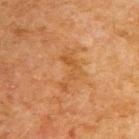Assessment:
Imaged during a routine full-body skin examination; the lesion was not biopsied and no histopathology is available.
Context:
A male subject, approximately 50 years of age. Cropped from a whole-body photographic skin survey; the tile spans about 15 mm. This is a cross-polarized tile. From the upper back. An algorithmic analysis of the crop reported an area of roughly 7.5 mm², an outline eccentricity of about 0.9 (0 = round, 1 = elongated), and two-axis asymmetry of about 0.65. It also reported a mean CIELAB color near L≈44 a*≈21 b*≈36 and about 5 CIELAB-L* units darker than the surrounding skin. It also reported an automated nevus-likeness rating near 0 out of 100 and a detector confidence of about 100 out of 100 that the crop contains a lesion.